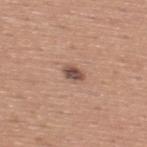Case summary:
– biopsy status — catalogued during a skin exam; not biopsied